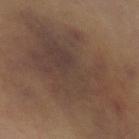Clinical impression: The lesion was photographed on a routine skin check and not biopsied; there is no pathology result.